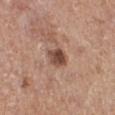Recorded during total-body skin imaging; not selected for excision or biopsy. The patient is a male roughly 75 years of age. The recorded lesion diameter is about 2.5 mm. The lesion is located on the right lower leg. Cropped from a whole-body photographic skin survey; the tile spans about 15 mm. This is a white-light tile.